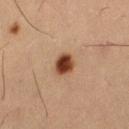biopsy status: imaged on a skin check; not biopsied
automated lesion analysis: a footprint of about 5 mm² and a symmetry-axis asymmetry near 0.2; a lesion color around L≈35 a*≈21 b*≈29 in CIELAB and a lesion–skin lightness drop of about 17; border irregularity of about 1.5 on a 0–10 scale; a lesion-detection confidence of about 100/100
tile lighting: cross-polarized illumination
anatomic site: the left thigh
patient: male, aged 53–57
imaging modality: ~15 mm tile from a whole-body skin photo
lesion size: ~2.5 mm (longest diameter)